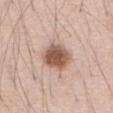Q: Was a biopsy performed?
A: total-body-photography surveillance lesion; no biopsy
Q: How was the tile lit?
A: white-light
Q: What are the patient's age and sex?
A: male, about 70 years old
Q: Lesion location?
A: the abdomen
Q: What is the lesion's diameter?
A: ≈4 mm
Q: Automated lesion metrics?
A: a mean CIELAB color near L≈56 a*≈20 b*≈27, about 16 CIELAB-L* units darker than the surrounding skin, and a normalized border contrast of about 10; a border-irregularity rating of about 1.5/10, internal color variation of about 4.5 on a 0–10 scale, and radial color variation of about 1; a nevus-likeness score of about 100/100
Q: What kind of image is this?
A: total-body-photography crop, ~15 mm field of view